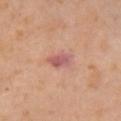Q: Was a biopsy performed?
A: total-body-photography surveillance lesion; no biopsy
Q: What are the patient's age and sex?
A: female, roughly 55 years of age
Q: Lesion size?
A: ~3 mm (longest diameter)
Q: What is the anatomic site?
A: the arm
Q: What lighting was used for the tile?
A: cross-polarized illumination
Q: How was this image acquired?
A: ~15 mm tile from a whole-body skin photo